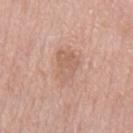From the mid back. A 15 mm close-up extracted from a 3D total-body photography capture. This is a white-light tile. Measured at roughly 4.5 mm in maximum diameter. A male patient, aged approximately 60.